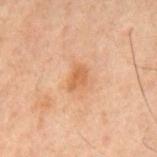<record>
  <biopsy_status>not biopsied; imaged during a skin examination</biopsy_status>
  <patient>
    <sex>male</sex>
    <age_approx>60</age_approx>
  </patient>
  <site>mid back</site>
  <automated_metrics>
    <cielab_L>47</cielab_L>
    <cielab_a>19</cielab_a>
    <cielab_b>31</cielab_b>
    <vs_skin_darker_L>7.0</vs_skin_darker_L>
    <vs_skin_contrast_norm>6.5</vs_skin_contrast_norm>
    <nevus_likeness_0_100>15</nevus_likeness_0_100>
  </automated_metrics>
  <lighting>cross-polarized</lighting>
  <image>
    <source>total-body photography crop</source>
    <field_of_view_mm>15</field_of_view_mm>
  </image>
  <lesion_size>
    <long_diameter_mm_approx>3.0</long_diameter_mm_approx>
  </lesion_size>
</record>